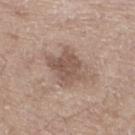Assessment: No biopsy was performed on this lesion — it was imaged during a full skin examination and was not determined to be concerning. Acquisition and patient details: A male patient aged 68–72. The lesion is on the leg. A lesion tile, about 15 mm wide, cut from a 3D total-body photograph. Imaged with white-light lighting. Measured at roughly 4.5 mm in maximum diameter.